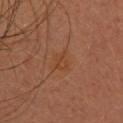Impression: The lesion was photographed on a routine skin check and not biopsied; there is no pathology result. Clinical summary: The patient is a female approximately 40 years of age. A close-up tile cropped from a whole-body skin photograph, about 15 mm across. This is a cross-polarized tile. The lesion is located on the back. The lesion-visualizer software estimated a border-irregularity rating of about 3.5/10 and peripheral color asymmetry of about 1. The recorded lesion diameter is about 2.5 mm.